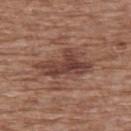Impression:
The lesion was photographed on a routine skin check and not biopsied; there is no pathology result.
Acquisition and patient details:
The lesion-visualizer software estimated a footprint of about 14 mm² and a symmetry-axis asymmetry near 0.5. The software also gave an average lesion color of about L≈42 a*≈21 b*≈24 (CIELAB) and a normalized border contrast of about 8.5. And it measured a classifier nevus-likeness of about 50/100 and lesion-presence confidence of about 100/100. Approximately 6.5 mm at its widest. A female subject, aged 73 to 77. On the upper back. This is a white-light tile. This image is a 15 mm lesion crop taken from a total-body photograph.